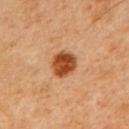Clinical impression: The lesion was tiled from a total-body skin photograph and was not biopsied. Context: A close-up tile cropped from a whole-body skin photograph, about 15 mm across. The subject is a male about 45 years old. Automated image analysis of the tile measured a border-irregularity rating of about 1.5/10, a within-lesion color-variation index near 4/10, and radial color variation of about 1.5. And it measured an automated nevus-likeness rating near 100 out of 100 and lesion-presence confidence of about 100/100. Captured under cross-polarized illumination. From the upper back.